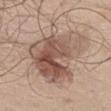Imaged during a routine full-body skin examination; the lesion was not biopsied and no histopathology is available.
A male subject about 55 years old.
Approximately 8.5 mm at its widest.
A lesion tile, about 15 mm wide, cut from a 3D total-body photograph.
An algorithmic analysis of the crop reported a mean CIELAB color near L≈54 a*≈17 b*≈25 and a normalized lesion–skin contrast near 8.5. And it measured a border-irregularity rating of about 5.5/10 and a peripheral color-asymmetry measure near 3.5.
The lesion is on the front of the torso.
The tile uses white-light illumination.